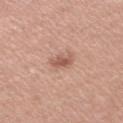Case summary:
- automated lesion analysis · an outline eccentricity of about 0.9 (0 = round, 1 = elongated) and two-axis asymmetry of about 0.25; a border-irregularity index near 3/10, internal color variation of about 2.5 on a 0–10 scale, and radial color variation of about 1
- body site · the arm
- patient · female, approximately 35 years of age
- image source · ~15 mm tile from a whole-body skin photo
- tile lighting · white-light illumination